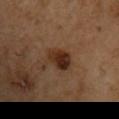Part of a total-body skin-imaging series; this lesion was reviewed on a skin check and was not flagged for biopsy. The lesion is located on the right upper arm. The patient is a male in their mid- to late 50s. This image is a 15 mm lesion crop taken from a total-body photograph. The total-body-photography lesion software estimated an average lesion color of about L≈28 a*≈18 b*≈27 (CIELAB), a lesion–skin lightness drop of about 11, and a normalized border contrast of about 10.5. The analysis additionally found an automated nevus-likeness rating near 95 out of 100 and a lesion-detection confidence of about 100/100. Captured under cross-polarized illumination. About 3.5 mm across.